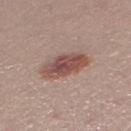Case summary:
• notes · catalogued during a skin exam; not biopsied
• lighting · white-light illumination
• patient · female, roughly 25 years of age
• site · the left thigh
• imaging modality · ~15 mm tile from a whole-body skin photo